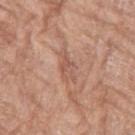{"biopsy_status": "not biopsied; imaged during a skin examination", "lighting": "white-light", "image": {"source": "total-body photography crop", "field_of_view_mm": 15}, "patient": {"sex": "female", "age_approx": 75}, "site": "left thigh", "automated_metrics": {"area_mm2_approx": 5.5, "eccentricity": 0.9, "shape_asymmetry": 0.45, "border_irregularity_0_10": 5.5, "peripheral_color_asymmetry": 1.0, "nevus_likeness_0_100": 0, "lesion_detection_confidence_0_100": 85}}